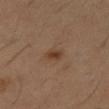notes: catalogued during a skin exam; not biopsied | subject: male, aged 58 to 62 | location: the right thigh | imaging modality: 15 mm crop, total-body photography | lighting: cross-polarized | lesion diameter: about 2 mm.